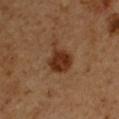| feature | finding |
|---|---|
| image source | ~15 mm crop, total-body skin-cancer survey |
| location | the right upper arm |
| patient | male, aged around 50 |